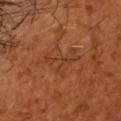Clinical impression:
Captured during whole-body skin photography for melanoma surveillance; the lesion was not biopsied.
Image and clinical context:
A region of skin cropped from a whole-body photographic capture, roughly 15 mm wide. The recorded lesion diameter is about 5 mm. The tile uses cross-polarized illumination. On the head or neck. A male subject, aged 48–52.